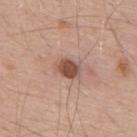{
  "biopsy_status": "not biopsied; imaged during a skin examination",
  "site": "upper back",
  "image": {
    "source": "total-body photography crop",
    "field_of_view_mm": 15
  },
  "patient": {
    "sex": "male",
    "age_approx": 55
  }
}